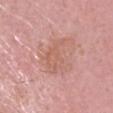Findings:
* follow-up: catalogued during a skin exam; not biopsied
* subject: male, aged 58–62
* diameter: about 6 mm
* tile lighting: white-light illumination
* anatomic site: the head or neck
* acquisition: 15 mm crop, total-body photography